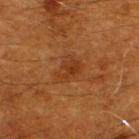Q: Is there a histopathology result?
A: catalogued during a skin exam; not biopsied
Q: What is the lesion's diameter?
A: about 3.5 mm
Q: Who is the patient?
A: male, aged 58–62
Q: What did automated image analysis measure?
A: a lesion area of about 6.5 mm² and a symmetry-axis asymmetry near 0.25; an average lesion color of about L≈35 a*≈24 b*≈36 (CIELAB), roughly 6 lightness units darker than nearby skin, and a normalized lesion–skin contrast near 6; border irregularity of about 2.5 on a 0–10 scale, internal color variation of about 3.5 on a 0–10 scale, and radial color variation of about 1
Q: What kind of image is this?
A: 15 mm crop, total-body photography
Q: Where on the body is the lesion?
A: the back
Q: Illumination type?
A: cross-polarized